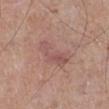The lesion was tiled from a total-body skin photograph and was not biopsied. A 15 mm crop from a total-body photograph taken for skin-cancer surveillance. The lesion is on the right lower leg. The subject is a male about 70 years old.